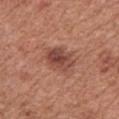{
  "biopsy_status": "not biopsied; imaged during a skin examination",
  "automated_metrics": {
    "area_mm2_approx": 9.0,
    "shape_asymmetry": 0.2,
    "cielab_L": 46,
    "cielab_a": 25,
    "cielab_b": 28,
    "border_irregularity_0_10": 2.5,
    "nevus_likeness_0_100": 65
  },
  "lesion_size": {
    "long_diameter_mm_approx": 4.0
  },
  "patient": {
    "sex": "male",
    "age_approx": 75
  },
  "image": {
    "source": "total-body photography crop",
    "field_of_view_mm": 15
  },
  "lighting": "white-light",
  "site": "chest"
}